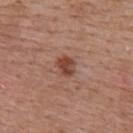Notes:
• follow-up — no biopsy performed (imaged during a skin exam)
• illumination — white-light illumination
• lesion diameter — ~2.5 mm (longest diameter)
• subject — female, roughly 50 years of age
• image-analysis metrics — an outline eccentricity of about 0.6 (0 = round, 1 = elongated) and two-axis asymmetry of about 0.2; an automated nevus-likeness rating near 75 out of 100
• anatomic site — the upper back
• acquisition — total-body-photography crop, ~15 mm field of view